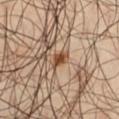Findings:
- biopsy status — imaged on a skin check; not biopsied
- subject — male, aged 58 to 62
- site — the left thigh
- lighting — cross-polarized
- acquisition — total-body-photography crop, ~15 mm field of view
- diameter — ~2.5 mm (longest diameter)
- TBP lesion metrics — a footprint of about 3 mm² and an eccentricity of roughly 0.8; an average lesion color of about L≈45 a*≈20 b*≈33 (CIELAB); border irregularity of about 3 on a 0–10 scale and peripheral color asymmetry of about 0.5; a classifier nevus-likeness of about 90/100 and a lesion-detection confidence of about 100/100